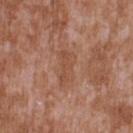Context:
On the upper back. A roughly 15 mm field-of-view crop from a total-body skin photograph. The tile uses white-light illumination. A male subject, in their mid- to late 40s. The recorded lesion diameter is about 4.5 mm.